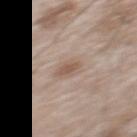follow-up: catalogued during a skin exam; not biopsied